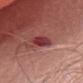<record>
<biopsy_status>not biopsied; imaged during a skin examination</biopsy_status>
<automated_metrics>
  <area_mm2_approx>5.5</area_mm2_approx>
  <eccentricity>0.75</eccentricity>
  <shape_asymmetry>0.2</shape_asymmetry>
  <cielab_L>38</cielab_L>
  <cielab_a>32</cielab_a>
  <cielab_b>21</cielab_b>
  <vs_skin_darker_L>12.0</vs_skin_darker_L>
  <border_irregularity_0_10>2.0</border_irregularity_0_10>
  <color_variation_0_10>4.5</color_variation_0_10>
  <peripheral_color_asymmetry>1.5</peripheral_color_asymmetry>
</automated_metrics>
<image>
  <source>total-body photography crop</source>
  <field_of_view_mm>15</field_of_view_mm>
</image>
<lesion_size>
  <long_diameter_mm_approx>3.0</long_diameter_mm_approx>
</lesion_size>
<site>head or neck</site>
<patient>
  <sex>female</sex>
  <age_approx>65</age_approx>
</patient>
<lighting>white-light</lighting>
</record>